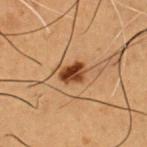A male patient, approximately 50 years of age.
The tile uses cross-polarized illumination.
The lesion is located on the chest.
Longest diameter approximately 3 mm.
A region of skin cropped from a whole-body photographic capture, roughly 15 mm wide.